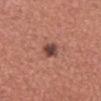<tbp_lesion>
  <biopsy_status>not biopsied; imaged during a skin examination</biopsy_status>
</tbp_lesion>